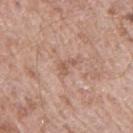follow-up: no biopsy performed (imaged during a skin exam); subject: male, about 55 years old; anatomic site: the right upper arm; image source: ~15 mm crop, total-body skin-cancer survey; lesion size: about 2.5 mm; automated metrics: an average lesion color of about L≈56 a*≈20 b*≈27 (CIELAB) and a lesion–skin lightness drop of about 8; tile lighting: white-light illumination.